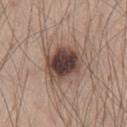image = 15 mm crop, total-body photography
subject = male, in their mid-40s
body site = the chest